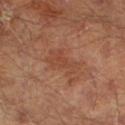Clinical impression: Imaged during a routine full-body skin examination; the lesion was not biopsied and no histopathology is available.